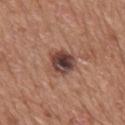Clinical impression: The lesion was tiled from a total-body skin photograph and was not biopsied. Background: The patient is a male in their mid-60s. Automated image analysis of the tile measured a shape-asymmetry score of about 0.2 (0 = symmetric). The analysis additionally found a mean CIELAB color near L≈43 a*≈20 b*≈24, roughly 14 lightness units darker than nearby skin, and a normalized border contrast of about 11. A region of skin cropped from a whole-body photographic capture, roughly 15 mm wide. The lesion's longest dimension is about 3.5 mm. This is a white-light tile. The lesion is located on the mid back.